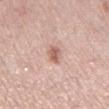No biopsy was performed on this lesion — it was imaged during a full skin examination and was not determined to be concerning.
This image is a 15 mm lesion crop taken from a total-body photograph.
On the left lower leg.
The lesion-visualizer software estimated an average lesion color of about L≈61 a*≈21 b*≈26 (CIELAB), a lesion–skin lightness drop of about 12, and a normalized border contrast of about 7.5.
The subject is a female aged 63 to 67.
Captured under white-light illumination.
The recorded lesion diameter is about 2.5 mm.